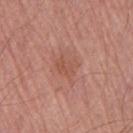Clinical impression:
No biopsy was performed on this lesion — it was imaged during a full skin examination and was not determined to be concerning.
Clinical summary:
Imaged with white-light lighting. The lesion's longest dimension is about 3 mm. A lesion tile, about 15 mm wide, cut from a 3D total-body photograph. A male patient roughly 65 years of age. On the arm.